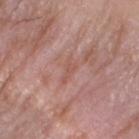Q: Was this lesion biopsied?
A: no biopsy performed (imaged during a skin exam)
Q: Lesion location?
A: the arm
Q: What kind of image is this?
A: 15 mm crop, total-body photography
Q: What are the patient's age and sex?
A: male, about 60 years old